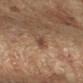This lesion was catalogued during total-body skin photography and was not selected for biopsy. From the right forearm. Approximately 3.5 mm at its widest. The subject is female. Cropped from a whole-body photographic skin survey; the tile spans about 15 mm.